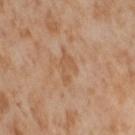{
  "biopsy_status": "not biopsied; imaged during a skin examination",
  "automated_metrics": {
    "eccentricity": 0.85,
    "shape_asymmetry": 0.5,
    "border_irregularity_0_10": 6.0,
    "color_variation_0_10": 1.0,
    "peripheral_color_asymmetry": 0.5
  },
  "site": "right thigh",
  "image": {
    "source": "total-body photography crop",
    "field_of_view_mm": 15
  },
  "patient": {
    "sex": "female",
    "age_approx": 55
  }
}